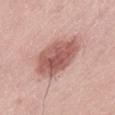follow-up = catalogued during a skin exam; not biopsied
automated metrics = an area of roughly 20 mm², an eccentricity of roughly 0.7, and a symmetry-axis asymmetry near 0.2; a mean CIELAB color near L≈57 a*≈24 b*≈25 and a lesion–skin lightness drop of about 14; peripheral color asymmetry of about 1.5; a nevus-likeness score of about 70/100 and a lesion-detection confidence of about 100/100
imaging modality = ~15 mm crop, total-body skin-cancer survey
lesion diameter = about 6 mm
subject = male, approximately 50 years of age
site = the left thigh
tile lighting = white-light